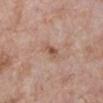Impression: Captured during whole-body skin photography for melanoma surveillance; the lesion was not biopsied. Background: A lesion tile, about 15 mm wide, cut from a 3D total-body photograph. Imaged with white-light lighting. A female subject about 70 years old. Located on the left lower leg.